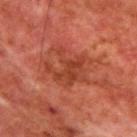Imaged during a routine full-body skin examination; the lesion was not biopsied and no histopathology is available. The lesion's longest dimension is about 5.5 mm. Cropped from a total-body skin-imaging series; the visible field is about 15 mm. On the upper back. Automated tile analysis of the lesion measured an eccentricity of roughly 0.55 and a shape-asymmetry score of about 0.5 (0 = symmetric). It also reported a mean CIELAB color near L≈39 a*≈29 b*≈31, a lesion–skin lightness drop of about 7, and a normalized border contrast of about 6. This is a cross-polarized tile. A male subject aged around 65.